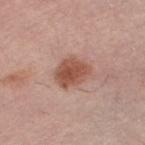The lesion was photographed on a routine skin check and not biopsied; there is no pathology result.
Approximately 4 mm at its widest.
A female patient, about 65 years old.
The lesion is located on the left thigh.
A roughly 15 mm field-of-view crop from a total-body skin photograph.